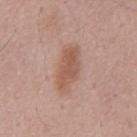Case summary:
- biopsy status: no biopsy performed (imaged during a skin exam)
- lesion diameter: about 5.5 mm
- lighting: white-light
- patient: male, aged 53 to 57
- acquisition: 15 mm crop, total-body photography
- image-analysis metrics: an average lesion color of about L≈56 a*≈21 b*≈28 (CIELAB), roughly 9 lightness units darker than nearby skin, and a normalized border contrast of about 7; a border-irregularity rating of about 3/10 and internal color variation of about 2.5 on a 0–10 scale; a lesion-detection confidence of about 100/100